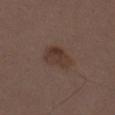Notes:
– biopsy status: catalogued during a skin exam; not biopsied
– acquisition: total-body-photography crop, ~15 mm field of view
– automated metrics: a footprint of about 8 mm² and an eccentricity of roughly 0.75; a mean CIELAB color near L≈35 a*≈16 b*≈22, roughly 7 lightness units darker than nearby skin, and a normalized border contrast of about 7; a within-lesion color-variation index near 3.5/10 and peripheral color asymmetry of about 1.5; an automated nevus-likeness rating near 55 out of 100 and a lesion-detection confidence of about 100/100
– size: ~4 mm (longest diameter)
– patient: female, about 40 years old
– tile lighting: white-light
– anatomic site: the left thigh